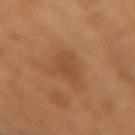Q: Is there a histopathology result?
A: imaged on a skin check; not biopsied
Q: What is the imaging modality?
A: total-body-photography crop, ~15 mm field of view
Q: Automated lesion metrics?
A: internal color variation of about 1 on a 0–10 scale and radial color variation of about 0.5
Q: What lighting was used for the tile?
A: cross-polarized illumination
Q: How large is the lesion?
A: about 3.5 mm
Q: Lesion location?
A: the arm
Q: What are the patient's age and sex?
A: female, about 55 years old